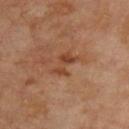<lesion>
<biopsy_status>not biopsied; imaged during a skin examination</biopsy_status>
<lesion_size>
  <long_diameter_mm_approx>3.5</long_diameter_mm_approx>
</lesion_size>
<automated_metrics>
  <cielab_L>43</cielab_L>
  <cielab_a>23</cielab_a>
  <cielab_b>32</cielab_b>
  <vs_skin_contrast_norm>6.5</vs_skin_contrast_norm>
  <color_variation_0_10>1.5</color_variation_0_10>
  <peripheral_color_asymmetry>0.5</peripheral_color_asymmetry>
  <nevus_likeness_0_100>0</nevus_likeness_0_100>
</automated_metrics>
<lighting>cross-polarized</lighting>
<image>
  <source>total-body photography crop</source>
  <field_of_view_mm>15</field_of_view_mm>
</image>
<patient>
  <sex>male</sex>
  <age_approx>65</age_approx>
</patient>
</lesion>